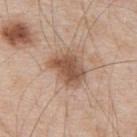Clinical impression:
The lesion was photographed on a routine skin check and not biopsied; there is no pathology result.
Image and clinical context:
A region of skin cropped from a whole-body photographic capture, roughly 15 mm wide. Located on the back. The lesion's longest dimension is about 4.5 mm. The subject is a male aged 48–52.